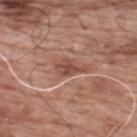Assessment: The lesion was tiled from a total-body skin photograph and was not biopsied. Clinical summary: From the upper back. The lesion-visualizer software estimated an area of roughly 4 mm² and an eccentricity of roughly 0.75. It also reported a mean CIELAB color near L≈48 a*≈23 b*≈27, about 10 CIELAB-L* units darker than the surrounding skin, and a lesion-to-skin contrast of about 7 (normalized; higher = more distinct). And it measured a peripheral color-asymmetry measure near 1. A male subject aged 58–62. The tile uses white-light illumination. Cropped from a total-body skin-imaging series; the visible field is about 15 mm.